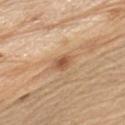Findings:
• biopsy status — catalogued during a skin exam; not biopsied
• lesion diameter — ~2.5 mm (longest diameter)
• tile lighting — white-light illumination
• imaging modality — 15 mm crop, total-body photography
• automated metrics — a lesion area of about 4 mm² and an outline eccentricity of about 0.7 (0 = round, 1 = elongated); a border-irregularity rating of about 1/10, a within-lesion color-variation index near 4/10, and a peripheral color-asymmetry measure near 1.5
• subject — male, about 70 years old
• body site — the arm